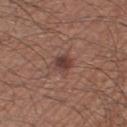Assessment:
Part of a total-body skin-imaging series; this lesion was reviewed on a skin check and was not flagged for biopsy.
Background:
A roughly 15 mm field-of-view crop from a total-body skin photograph. The recorded lesion diameter is about 2.5 mm. Automated tile analysis of the lesion measured a border-irregularity index near 2.5/10, a within-lesion color-variation index near 3/10, and radial color variation of about 1. On the right thigh. The patient is a male in their 50s. Captured under white-light illumination.